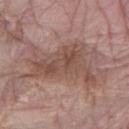Clinical impression: No biopsy was performed on this lesion — it was imaged during a full skin examination and was not determined to be concerning. Background: The tile uses white-light illumination. The lesion's longest dimension is about 9.5 mm. A female patient aged 63–67. Located on the right forearm. The total-body-photography lesion software estimated a peripheral color-asymmetry measure near 2. A close-up tile cropped from a whole-body skin photograph, about 15 mm across.